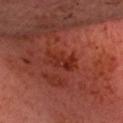follow-up — catalogued during a skin exam; not biopsied
anatomic site — the head or neck
lesion size — ≈3.5 mm
image source — ~15 mm tile from a whole-body skin photo
patient — male, aged around 60